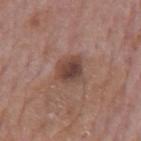Notes:
* notes — total-body-photography surveillance lesion; no biopsy
* subject — male, aged around 75
* image-analysis metrics — a lesion area of about 8 mm², a shape eccentricity near 0.45, and two-axis asymmetry of about 0.15; border irregularity of about 1.5 on a 0–10 scale, internal color variation of about 5 on a 0–10 scale, and peripheral color asymmetry of about 1.5
* anatomic site — the mid back
* lighting — white-light
* imaging modality — ~15 mm crop, total-body skin-cancer survey
* diameter — ~3 mm (longest diameter)